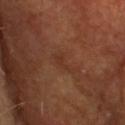Q: How was the tile lit?
A: cross-polarized
Q: What is the lesion's diameter?
A: ~2.5 mm (longest diameter)
Q: What did automated image analysis measure?
A: a lesion–skin lightness drop of about 4; a border-irregularity index near 6/10, a color-variation rating of about 0/10, and peripheral color asymmetry of about 0; a classifier nevus-likeness of about 0/100
Q: What kind of image is this?
A: ~15 mm crop, total-body skin-cancer survey
Q: What is the anatomic site?
A: the head or neck
Q: What are the patient's age and sex?
A: male, roughly 70 years of age